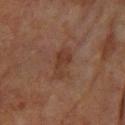This lesion was catalogued during total-body skin photography and was not selected for biopsy.
Automated tile analysis of the lesion measured a lesion color around L≈31 a*≈18 b*≈25 in CIELAB and a lesion-to-skin contrast of about 6.5 (normalized; higher = more distinct). The software also gave a peripheral color-asymmetry measure near 0.5.
The lesion is located on the right forearm.
A female subject aged around 80.
Cropped from a whole-body photographic skin survey; the tile spans about 15 mm.
Imaged with cross-polarized lighting.
The lesion's longest dimension is about 3.5 mm.